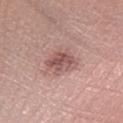Recorded during total-body skin imaging; not selected for excision or biopsy. Located on the arm. A roughly 15 mm field-of-view crop from a total-body skin photograph. A female subject in their mid- to late 30s. The recorded lesion diameter is about 4 mm.